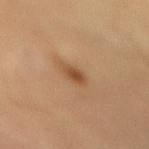{
  "biopsy_status": "not biopsied; imaged during a skin examination",
  "lighting": "cross-polarized",
  "image": {
    "source": "total-body photography crop",
    "field_of_view_mm": 15
  },
  "site": "mid back",
  "patient": {
    "sex": "male",
    "age_approx": 55
  },
  "lesion_size": {
    "long_diameter_mm_approx": 3.0
  },
  "automated_metrics": {
    "area_mm2_approx": 3.5,
    "shape_asymmetry": 0.25,
    "vs_skin_darker_L": 10.0,
    "vs_skin_contrast_norm": 7.5
  }
}